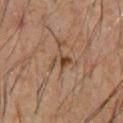This lesion was catalogued during total-body skin photography and was not selected for biopsy. The lesion is on the chest. A region of skin cropped from a whole-body photographic capture, roughly 15 mm wide. The tile uses cross-polarized illumination. A male subject, aged 58 to 62.